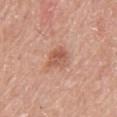biopsy status = total-body-photography surveillance lesion; no biopsy | diameter = about 3 mm | imaging modality = ~15 mm crop, total-body skin-cancer survey | patient = male, approximately 60 years of age | image-analysis metrics = an area of roughly 6.5 mm² and an eccentricity of roughly 0.55; a mean CIELAB color near L≈57 a*≈24 b*≈30, about 9 CIELAB-L* units darker than the surrounding skin, and a normalized lesion–skin contrast near 6.5; a border-irregularity index near 2.5/10, internal color variation of about 4 on a 0–10 scale, and peripheral color asymmetry of about 1.5; a classifier nevus-likeness of about 15/100 and a detector confidence of about 100 out of 100 that the crop contains a lesion | illumination = white-light illumination | location = the upper back.